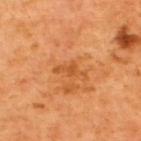Captured during whole-body skin photography for melanoma surveillance; the lesion was not biopsied.
The subject is a male about 65 years old.
A roughly 15 mm field-of-view crop from a total-body skin photograph.
On the back.
About 3.5 mm across.